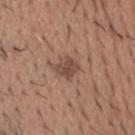The lesion is on the head or neck. This is a white-light tile. Measured at roughly 3 mm in maximum diameter. A male subject aged around 60. Cropped from a total-body skin-imaging series; the visible field is about 15 mm.